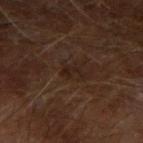Case summary:
– follow-up · total-body-photography surveillance lesion; no biopsy
– image · 15 mm crop, total-body photography
– body site · the left forearm
– subject · male, aged 63 to 67
– illumination · cross-polarized illumination
– automated metrics · an outline eccentricity of about 0.9 (0 = round, 1 = elongated) and a shape-asymmetry score of about 0.45 (0 = symmetric); an average lesion color of about L≈19 a*≈15 b*≈20 (CIELAB) and roughly 5 lightness units darker than nearby skin; a border-irregularity rating of about 5.5/10, internal color variation of about 0 on a 0–10 scale, and peripheral color asymmetry of about 0; a classifier nevus-likeness of about 0/100
– lesion diameter · ≈2.5 mm